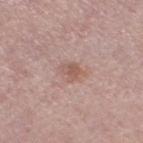| key | value |
|---|---|
| notes | no biopsy performed (imaged during a skin exam) |
| subject | female, aged approximately 50 |
| diameter | ≈2.5 mm |
| tile lighting | white-light |
| acquisition | ~15 mm crop, total-body skin-cancer survey |
| site | the leg |